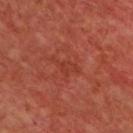Assessment: Recorded during total-body skin imaging; not selected for excision or biopsy. Background: A male patient aged approximately 60. Imaged with cross-polarized lighting. Longest diameter approximately 4 mm. The total-body-photography lesion software estimated a footprint of about 4.5 mm², a shape eccentricity near 0.9, and a shape-asymmetry score of about 0.55 (0 = symmetric). The software also gave an average lesion color of about L≈37 a*≈31 b*≈31 (CIELAB), a lesion–skin lightness drop of about 5, and a normalized lesion–skin contrast near 5. The software also gave a border-irregularity rating of about 6.5/10, a within-lesion color-variation index near 1.5/10, and radial color variation of about 0.5. The software also gave a nevus-likeness score of about 0/100 and a detector confidence of about 100 out of 100 that the crop contains a lesion. The lesion is on the chest. A lesion tile, about 15 mm wide, cut from a 3D total-body photograph.